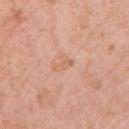The total-body-photography lesion software estimated an outline eccentricity of about 0.9 (0 = round, 1 = elongated) and a symmetry-axis asymmetry near 0.45. It also reported a lesion color around L≈63 a*≈24 b*≈34 in CIELAB and about 6 CIELAB-L* units darker than the surrounding skin. The analysis additionally found a within-lesion color-variation index near 0/10 and a peripheral color-asymmetry measure near 0. Located on the left upper arm. Imaged with white-light lighting. A female subject, aged around 70. A lesion tile, about 15 mm wide, cut from a 3D total-body photograph.